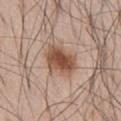The lesion was photographed on a routine skin check and not biopsied; there is no pathology result. An algorithmic analysis of the crop reported a classifier nevus-likeness of about 95/100. A close-up tile cropped from a whole-body skin photograph, about 15 mm across. The patient is a male aged 68 to 72. The tile uses white-light illumination. From the abdomen.